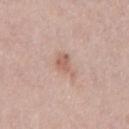Q: Is there a histopathology result?
A: imaged on a skin check; not biopsied
Q: Lesion location?
A: the mid back
Q: Lesion size?
A: about 3.5 mm
Q: Patient demographics?
A: male, about 40 years old
Q: What kind of image is this?
A: total-body-photography crop, ~15 mm field of view